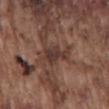Impression: Captured during whole-body skin photography for melanoma surveillance; the lesion was not biopsied. Image and clinical context: The lesion is located on the mid back. A male subject aged approximately 75. A 15 mm crop from a total-body photograph taken for skin-cancer surveillance.